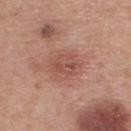Assessment: This lesion was catalogued during total-body skin photography and was not selected for biopsy. Acquisition and patient details: The recorded lesion diameter is about 3 mm. Captured under white-light illumination. A male patient in their mid-60s. A 15 mm crop from a total-body photograph taken for skin-cancer surveillance. On the upper back.